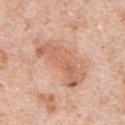* notes: total-body-photography surveillance lesion; no biopsy
* subject: male, about 80 years old
* imaging modality: total-body-photography crop, ~15 mm field of view
* site: the chest